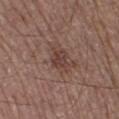  biopsy_status: not biopsied; imaged during a skin examination
  lighting: white-light
  site: leg
  patient:
    sex: male
    age_approx: 65
  lesion_size:
    long_diameter_mm_approx: 2.5
  automated_metrics:
    border_irregularity_0_10: 2.5
    color_variation_0_10: 2.5
    peripheral_color_asymmetry: 1.0
    nevus_likeness_0_100: 0
  image:
    source: total-body photography crop
    field_of_view_mm: 15